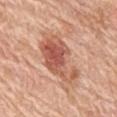notes: imaged on a skin check; not biopsied
location: the mid back
diameter: about 7 mm
imaging modality: 15 mm crop, total-body photography
image-analysis metrics: an area of roughly 19 mm², an eccentricity of roughly 0.85, and a shape-asymmetry score of about 0.35 (0 = symmetric); roughly 12 lightness units darker than nearby skin and a normalized border contrast of about 8; a border-irregularity rating of about 4.5/10 and a color-variation rating of about 6.5/10; a nevus-likeness score of about 85/100 and a detector confidence of about 100 out of 100 that the crop contains a lesion
subject: female, approximately 65 years of age